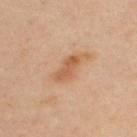Clinical impression: Imaged during a routine full-body skin examination; the lesion was not biopsied and no histopathology is available. Acquisition and patient details: A male subject, in their mid-30s. An algorithmic analysis of the crop reported an area of roughly 7 mm². The analysis additionally found a border-irregularity index near 4.5/10, a within-lesion color-variation index near 4/10, and radial color variation of about 1.5. The lesion is located on the upper back. A roughly 15 mm field-of-view crop from a total-body skin photograph. Approximately 4.5 mm at its widest. Captured under cross-polarized illumination.